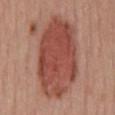The lesion was tiled from a total-body skin photograph and was not biopsied.
Imaged with white-light lighting.
This image is a 15 mm lesion crop taken from a total-body photograph.
A female patient, aged 53 to 57.
The lesion is on the mid back.
Measured at roughly 10.5 mm in maximum diameter.